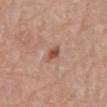Assessment:
The lesion was photographed on a routine skin check and not biopsied; there is no pathology result.
Acquisition and patient details:
The lesion's longest dimension is about 2.5 mm. Automated image analysis of the tile measured an average lesion color of about L≈51 a*≈22 b*≈28 (CIELAB), a lesion–skin lightness drop of about 12, and a normalized lesion–skin contrast near 8.5. The software also gave a border-irregularity rating of about 3.5/10, a color-variation rating of about 2.5/10, and a peripheral color-asymmetry measure near 1. And it measured a classifier nevus-likeness of about 80/100. A male subject aged approximately 80. A 15 mm close-up tile from a total-body photography series done for melanoma screening. The tile uses white-light illumination. Located on the mid back.